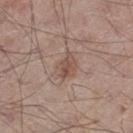The total-body-photography lesion software estimated a lesion area of about 5.5 mm² and two-axis asymmetry of about 0.25. And it measured a lesion color around L≈51 a*≈18 b*≈25 in CIELAB, a lesion–skin lightness drop of about 8, and a normalized lesion–skin contrast near 6. The analysis additionally found radial color variation of about 1.5. The lesion's longest dimension is about 3 mm. The patient is a male aged 53 to 57. The lesion is on the left thigh. Imaged with white-light lighting. A roughly 15 mm field-of-view crop from a total-body skin photograph.